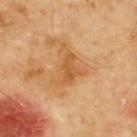subject: male, approximately 70 years of age; location: the upper back; illumination: cross-polarized illumination; image-analysis metrics: an area of roughly 8.5 mm² and two-axis asymmetry of about 0.5; size: ≈5 mm; image: 15 mm crop, total-body photography.